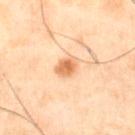Clinical impression: Captured during whole-body skin photography for melanoma surveillance; the lesion was not biopsied. Clinical summary: The patient is a male about 50 years old. A region of skin cropped from a whole-body photographic capture, roughly 15 mm wide. Captured under cross-polarized illumination. Located on the front of the torso. Automated image analysis of the tile measured a shape eccentricity near 0.7 and a symmetry-axis asymmetry near 0.2. It also reported a lesion color around L≈53 a*≈19 b*≈33 in CIELAB and a lesion–skin lightness drop of about 10. The lesion's longest dimension is about 3 mm.